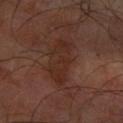Captured during whole-body skin photography for melanoma surveillance; the lesion was not biopsied. Cropped from a whole-body photographic skin survey; the tile spans about 15 mm. The subject is a male about 65 years old. The total-body-photography lesion software estimated a lesion color around L≈25 a*≈18 b*≈23 in CIELAB, a lesion–skin lightness drop of about 6, and a normalized border contrast of about 6.5. The analysis additionally found a nevus-likeness score of about 0/100 and a lesion-detection confidence of about 95/100. Imaged with cross-polarized lighting. On the left forearm.